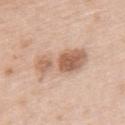| field | value |
|---|---|
| biopsy status | total-body-photography surveillance lesion; no biopsy |
| site | the left upper arm |
| lighting | white-light illumination |
| diameter | about 6.5 mm |
| image | total-body-photography crop, ~15 mm field of view |
| subject | female, approximately 65 years of age |